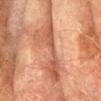- biopsy status · no biopsy performed (imaged during a skin exam)
- automated metrics · a lesion area of about 15 mm², an eccentricity of roughly 0.95, and a shape-asymmetry score of about 0.55 (0 = symmetric); a border-irregularity rating of about 9/10, a color-variation rating of about 3.5/10, and radial color variation of about 1; a classifier nevus-likeness of about 0/100 and lesion-presence confidence of about 65/100
- imaging modality · total-body-photography crop, ~15 mm field of view
- subject · male, approximately 75 years of age
- site · the right forearm
- lesion size · ~8 mm (longest diameter)